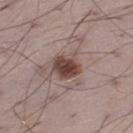| key | value |
|---|---|
| follow-up | imaged on a skin check; not biopsied |
| automated lesion analysis | border irregularity of about 1.5 on a 0–10 scale and a within-lesion color-variation index near 4.5/10 |
| illumination | white-light illumination |
| patient | male, aged 68 to 72 |
| imaging modality | 15 mm crop, total-body photography |
| body site | the left thigh |
| diameter | ≈3.5 mm |